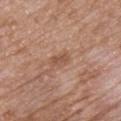Part of a total-body skin-imaging series; this lesion was reviewed on a skin check and was not flagged for biopsy.
The lesion-visualizer software estimated a lesion area of about 4.5 mm² and two-axis asymmetry of about 0.25. The software also gave an average lesion color of about L≈53 a*≈20 b*≈30 (CIELAB) and a lesion–skin lightness drop of about 8. The software also gave a classifier nevus-likeness of about 10/100.
Approximately 3 mm at its widest.
A male patient aged 53–57.
The tile uses white-light illumination.
This image is a 15 mm lesion crop taken from a total-body photograph.
The lesion is located on the front of the torso.